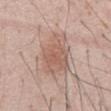Clinical impression:
Captured during whole-body skin photography for melanoma surveillance; the lesion was not biopsied.
Image and clinical context:
The lesion is on the abdomen. An algorithmic analysis of the crop reported an eccentricity of roughly 0.85. The analysis additionally found an average lesion color of about L≈58 a*≈19 b*≈26 (CIELAB) and a normalized border contrast of about 6.5. The analysis additionally found a border-irregularity rating of about 3/10, a within-lesion color-variation index near 3/10, and peripheral color asymmetry of about 1. The software also gave a nevus-likeness score of about 70/100 and a detector confidence of about 100 out of 100 that the crop contains a lesion. A close-up tile cropped from a whole-body skin photograph, about 15 mm across. Measured at roughly 6 mm in maximum diameter. Captured under white-light illumination. A male subject, approximately 55 years of age.